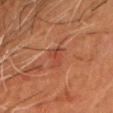Q: Automated lesion metrics?
A: an average lesion color of about L≈38 a*≈25 b*≈28 (CIELAB) and roughly 6 lightness units darker than nearby skin; border irregularity of about 4.5 on a 0–10 scale and peripheral color asymmetry of about 1
Q: What kind of image is this?
A: total-body-photography crop, ~15 mm field of view
Q: Who is the patient?
A: male, approximately 60 years of age
Q: What is the lesion's diameter?
A: ~3 mm (longest diameter)
Q: How was the tile lit?
A: cross-polarized illumination
Q: Lesion location?
A: the head or neck